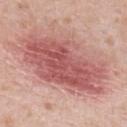| feature | finding |
|---|---|
| biopsy status | catalogued during a skin exam; not biopsied |